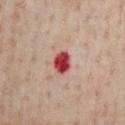  biopsy_status: not biopsied; imaged during a skin examination
  patient:
    sex: male
    age_approx: 65
  image:
    source: total-body photography crop
    field_of_view_mm: 15
  site: chest